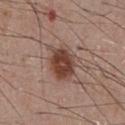  biopsy_status: not biopsied; imaged during a skin examination
  image:
    source: total-body photography crop
    field_of_view_mm: 15
  site: front of the torso
  patient:
    sex: male
    age_approx: 55